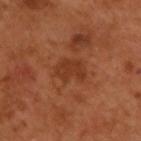Findings:
• notes — no biopsy performed (imaged during a skin exam)
• lesion diameter — ≈3.5 mm
• image-analysis metrics — a border-irregularity rating of about 2.5/10 and a color-variation rating of about 2/10; an automated nevus-likeness rating near 0 out of 100 and lesion-presence confidence of about 100/100
• imaging modality — total-body-photography crop, ~15 mm field of view
• body site — the upper back
• illumination — cross-polarized
• patient — male, about 50 years old